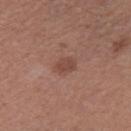  biopsy_status: not biopsied; imaged during a skin examination
  patient:
    sex: female
    age_approx: 40
  lesion_size:
    long_diameter_mm_approx: 2.5
  lighting: white-light
  site: right thigh
  image:
    source: total-body photography crop
    field_of_view_mm: 15